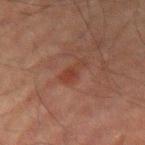notes = total-body-photography surveillance lesion; no biopsy
patient = male, aged approximately 70
anatomic site = the arm
lighting = cross-polarized illumination
lesion size = ≈3.5 mm
image source = ~15 mm crop, total-body skin-cancer survey